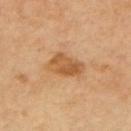biopsy_status: not biopsied; imaged during a skin examination
image:
  source: total-body photography crop
  field_of_view_mm: 15
lighting: cross-polarized
patient:
  sex: female
  age_approx: 65
automated_metrics:
  area_mm2_approx: 9.0
  eccentricity: 0.8
  shape_asymmetry: 0.3
  vs_skin_contrast_norm: 7.5
  border_irregularity_0_10: 3.0
  color_variation_0_10: 4.5
  peripheral_color_asymmetry: 2.0
  nevus_likeness_0_100: 15
  lesion_detection_confidence_0_100: 100
lesion_size:
  long_diameter_mm_approx: 4.0
site: upper back